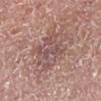| field | value |
|---|---|
| biopsy status | imaged on a skin check; not biopsied |
| subject | male, aged 53 to 57 |
| lesion diameter | about 4.5 mm |
| imaging modality | ~15 mm tile from a whole-body skin photo |
| automated lesion analysis | a lesion area of about 14 mm², an eccentricity of roughly 0.5, and a shape-asymmetry score of about 0.3 (0 = symmetric); a lesion color around L≈52 a*≈19 b*≈21 in CIELAB and a lesion-to-skin contrast of about 5.5 (normalized; higher = more distinct); a border-irregularity rating of about 5/10 and radial color variation of about 1.5; a classifier nevus-likeness of about 0/100 and a detector confidence of about 80 out of 100 that the crop contains a lesion |
| body site | the left lower leg |
| lighting | white-light illumination |